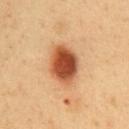Clinical impression: The lesion was photographed on a routine skin check and not biopsied; there is no pathology result.